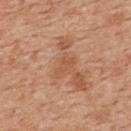  biopsy_status: not biopsied; imaged during a skin examination
  site: back
  patient:
    sex: male
    age_approx: 60
  lesion_size:
    long_diameter_mm_approx: 2.5
  automated_metrics:
    cielab_L: 54
    cielab_a: 23
    cielab_b: 34
    vs_skin_darker_L: 6.0
    vs_skin_contrast_norm: 5.0
    border_irregularity_0_10: 3.0
    color_variation_0_10: 1.0
    peripheral_color_asymmetry: 0.0
    nevus_likeness_0_100: 0
  lighting: white-light
  image:
    source: total-body photography crop
    field_of_view_mm: 15